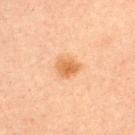Clinical impression: The lesion was photographed on a routine skin check and not biopsied; there is no pathology result. Background: A 15 mm close-up extracted from a 3D total-body photography capture. About 2.5 mm across. A male patient aged approximately 30. Located on the upper back. This is a cross-polarized tile.